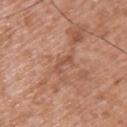This lesion was catalogued during total-body skin photography and was not selected for biopsy. The lesion's longest dimension is about 3 mm. A 15 mm crop from a total-body photograph taken for skin-cancer surveillance. A male patient, aged 48 to 52. The lesion is on the upper back. Imaged with white-light lighting.